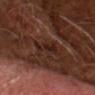Clinical impression:
Part of a total-body skin-imaging series; this lesion was reviewed on a skin check and was not flagged for biopsy.
Acquisition and patient details:
An algorithmic analysis of the crop reported a within-lesion color-variation index near 2.5/10 and a peripheral color-asymmetry measure near 0.5. And it measured a classifier nevus-likeness of about 0/100 and a detector confidence of about 65 out of 100 that the crop contains a lesion. The patient is a male aged approximately 65. A close-up tile cropped from a whole-body skin photograph, about 15 mm across. Captured under cross-polarized illumination.